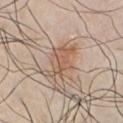The lesion was tiled from a total-body skin photograph and was not biopsied. Longest diameter approximately 5 mm. Located on the chest. The total-body-photography lesion software estimated a shape eccentricity near 0.85 and a shape-asymmetry score of about 0.65 (0 = symmetric). The patient is a male aged approximately 45. A 15 mm close-up tile from a total-body photography series done for melanoma screening.